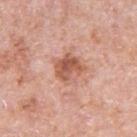Clinical impression: The lesion was photographed on a routine skin check and not biopsied; there is no pathology result. Image and clinical context: Located on the left upper arm. A male subject, aged 78–82. Cropped from a total-body skin-imaging series; the visible field is about 15 mm.